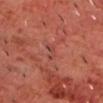Assessment: The lesion was photographed on a routine skin check and not biopsied; there is no pathology result. Acquisition and patient details: The lesion is located on the chest. A male patient, aged approximately 70. The tile uses cross-polarized illumination. Longest diameter approximately 3 mm. A roughly 15 mm field-of-view crop from a total-body skin photograph.